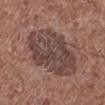The lesion was photographed on a routine skin check and not biopsied; there is no pathology result. Approximately 8 mm at its widest. The subject is a female about 50 years old. The lesion-visualizer software estimated a lesion area of about 34 mm² and an outline eccentricity of about 0.75 (0 = round, 1 = elongated). The software also gave a mean CIELAB color near L≈43 a*≈17 b*≈20, about 10 CIELAB-L* units darker than the surrounding skin, and a normalized border contrast of about 8.5. The software also gave an automated nevus-likeness rating near 15 out of 100 and lesion-presence confidence of about 100/100. The lesion is on the left lower leg. Captured under white-light illumination. Cropped from a total-body skin-imaging series; the visible field is about 15 mm.